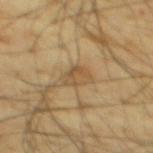follow-up: no biopsy performed (imaged during a skin exam); subject: male, aged 63 to 67; body site: the mid back; diameter: ~3 mm (longest diameter); acquisition: 15 mm crop, total-body photography.